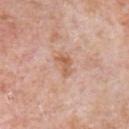Captured during whole-body skin photography for melanoma surveillance; the lesion was not biopsied. A close-up tile cropped from a whole-body skin photograph, about 15 mm across. A male patient, aged approximately 80. On the chest. Automated tile analysis of the lesion measured a lesion area of about 3.5 mm² and a symmetry-axis asymmetry near 0.5. The analysis additionally found a nevus-likeness score of about 0/100.